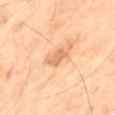The lesion was photographed on a routine skin check and not biopsied; there is no pathology result. The lesion is on the left thigh. The lesion-visualizer software estimated a mean CIELAB color near L≈69 a*≈23 b*≈41, about 10 CIELAB-L* units darker than the surrounding skin, and a normalized border contrast of about 6. The analysis additionally found a lesion-detection confidence of about 100/100. A 15 mm close-up tile from a total-body photography series done for melanoma screening. The patient is a male in their 70s.